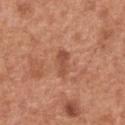follow-up: total-body-photography surveillance lesion; no biopsy
TBP lesion metrics: a footprint of about 4 mm² and an outline eccentricity of about 0.9 (0 = round, 1 = elongated); a lesion color around L≈51 a*≈26 b*≈32 in CIELAB, a lesion–skin lightness drop of about 9, and a normalized border contrast of about 6.5; an automated nevus-likeness rating near 0 out of 100 and lesion-presence confidence of about 100/100
illumination: white-light
acquisition: total-body-photography crop, ~15 mm field of view
lesion size: ~3 mm (longest diameter)
location: the front of the torso
subject: male, aged 63–67